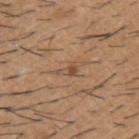Impression: The lesion was photographed on a routine skin check and not biopsied; there is no pathology result. Background: A 15 mm crop from a total-body photograph taken for skin-cancer surveillance. The tile uses white-light illumination. The lesion's longest dimension is about 3 mm. A male subject, aged 58 to 62. Automated tile analysis of the lesion measured an area of roughly 3 mm², an outline eccentricity of about 0.85 (0 = round, 1 = elongated), and two-axis asymmetry of about 0.55. The software also gave a mean CIELAB color near L≈50 a*≈18 b*≈30 and a lesion–skin lightness drop of about 8. The lesion is located on the upper back.